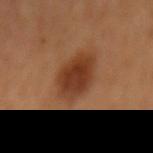The lesion was tiled from a total-body skin photograph and was not biopsied. Imaged with cross-polarized lighting. The patient is female. A lesion tile, about 15 mm wide, cut from a 3D total-body photograph. Longest diameter approximately 5.5 mm. On the mid back.